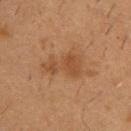notes: imaged on a skin check; not biopsied | patient: male, aged 53 to 57 | illumination: cross-polarized illumination | image source: ~15 mm tile from a whole-body skin photo | site: the chest | size: ≈4.5 mm.